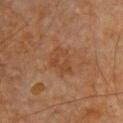notes: imaged on a skin check; not biopsied
automated lesion analysis: a lesion-detection confidence of about 100/100
diameter: ≈4 mm
body site: the chest
tile lighting: cross-polarized
subject: male, approximately 65 years of age
image: 15 mm crop, total-body photography